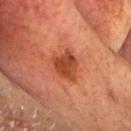- size — ~4 mm (longest diameter)
- subject — male, aged around 60
- anatomic site — the head or neck
- acquisition — 15 mm crop, total-body photography
- automated lesion analysis — a mean CIELAB color near L≈46 a*≈31 b*≈38; a border-irregularity index near 3/10, internal color variation of about 4.5 on a 0–10 scale, and radial color variation of about 1.5